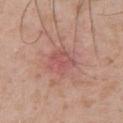workup — no biopsy performed (imaged during a skin exam) | patient — male, in their mid-50s | imaging modality — ~15 mm tile from a whole-body skin photo | automated metrics — a within-lesion color-variation index near 3/10 and peripheral color asymmetry of about 1; an automated nevus-likeness rating near 0 out of 100 | tile lighting — white-light illumination | lesion size — ~3.5 mm (longest diameter) | site — the upper back.